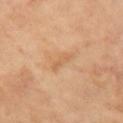biopsy status = total-body-photography surveillance lesion; no biopsy
size = about 3 mm
anatomic site = the left upper arm
image = 15 mm crop, total-body photography
subject = female, aged 73 to 77
illumination = cross-polarized illumination